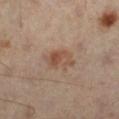The lesion was tiled from a total-body skin photograph and was not biopsied. Captured under cross-polarized illumination. Longest diameter approximately 4 mm. The subject is a male approximately 50 years of age. This image is a 15 mm lesion crop taken from a total-body photograph. The lesion is on the left leg.